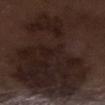Findings:
* workup — catalogued during a skin exam; not biopsied
* tile lighting — white-light
* lesion diameter — ~16 mm (longest diameter)
* imaging modality — ~15 mm tile from a whole-body skin photo
* location — the right lower leg
* subject — male, in their 70s